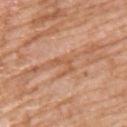workup: no biopsy performed (imaged during a skin exam)
size: ≈3 mm
acquisition: total-body-photography crop, ~15 mm field of view
location: the upper back
patient: male, in their mid- to late 70s
illumination: white-light